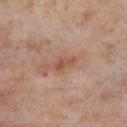Findings:
• biopsy status: imaged on a skin check; not biopsied
• location: the leg
• image: 15 mm crop, total-body photography
• diameter: about 2.5 mm
• subject: female, in their mid-50s
• lighting: cross-polarized